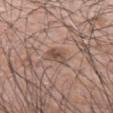Clinical impression:
Captured during whole-body skin photography for melanoma surveillance; the lesion was not biopsied.
Background:
The patient is a male in their mid-70s. The recorded lesion diameter is about 3 mm. The tile uses white-light illumination. A 15 mm crop from a total-body photograph taken for skin-cancer surveillance. The lesion is located on the left forearm.